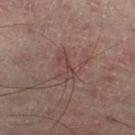{
  "biopsy_status": "not biopsied; imaged during a skin examination",
  "patient": {
    "sex": "male",
    "age_approx": 60
  },
  "lesion_size": {
    "long_diameter_mm_approx": 3.0
  },
  "site": "right lower leg",
  "image": {
    "source": "total-body photography crop",
    "field_of_view_mm": 15
  }
}